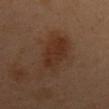Clinical impression: Captured during whole-body skin photography for melanoma surveillance; the lesion was not biopsied. Background: Imaged with cross-polarized lighting. A roughly 15 mm field-of-view crop from a total-body skin photograph. The patient is a male about 50 years old. On the mid back.